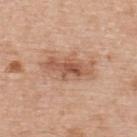  biopsy_status: not biopsied; imaged during a skin examination
  lighting: white-light
  site: upper back
  lesion_size:
    long_diameter_mm_approx: 6.0
  patient:
    sex: male
    age_approx: 70
  image:
    source: total-body photography crop
    field_of_view_mm: 15